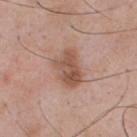- follow-up · catalogued during a skin exam; not biopsied
- patient · male, in their mid- to late 50s
- size · ~4.5 mm (longest diameter)
- location · the chest
- image source · ~15 mm crop, total-body skin-cancer survey
- tile lighting · white-light